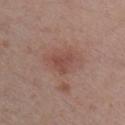Notes:
* biopsy status · total-body-photography surveillance lesion; no biopsy
* location · the right upper arm
* subject · male, aged around 55
* diameter · ~2.5 mm (longest diameter)
* lighting · white-light illumination
* automated metrics · a lesion area of about 4 mm², an outline eccentricity of about 0.35 (0 = round, 1 = elongated), and a shape-asymmetry score of about 0.5 (0 = symmetric); roughly 7 lightness units darker than nearby skin
* image · ~15 mm tile from a whole-body skin photo